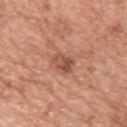This lesion was catalogued during total-body skin photography and was not selected for biopsy. A female subject, aged 53–57. Located on the upper back. A 15 mm close-up tile from a total-body photography series done for melanoma screening.